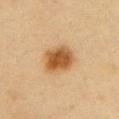{
  "biopsy_status": "not biopsied; imaged during a skin examination",
  "patient": {
    "sex": "female",
    "age_approx": 55
  },
  "site": "chest",
  "image": {
    "source": "total-body photography crop",
    "field_of_view_mm": 15
  }
}